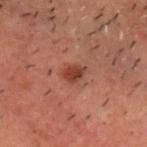No biopsy was performed on this lesion — it was imaged during a full skin examination and was not determined to be concerning.
The patient is a male about 50 years old.
A region of skin cropped from a whole-body photographic capture, roughly 15 mm wide.
This is a cross-polarized tile.
On the head or neck.
Longest diameter approximately 3 mm.